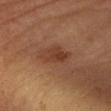Assessment: Recorded during total-body skin imaging; not selected for excision or biopsy. Context: Automated image analysis of the tile measured an average lesion color of about L≈36 a*≈20 b*≈28 (CIELAB) and about 7 CIELAB-L* units darker than the surrounding skin. The software also gave a border-irregularity rating of about 2.5/10, internal color variation of about 4 on a 0–10 scale, and a peripheral color-asymmetry measure near 1.5. And it measured a nevus-likeness score of about 45/100. The patient is a male aged around 60. This is a cross-polarized tile. A region of skin cropped from a whole-body photographic capture, roughly 15 mm wide. The lesion is located on the left forearm.